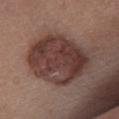* workup · catalogued during a skin exam; not biopsied
* image source · total-body-photography crop, ~15 mm field of view
* subject · male, approximately 55 years of age
* location · the leg